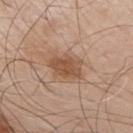| feature | finding |
|---|---|
| anatomic site | the chest |
| subject | male, aged 78 to 82 |
| imaging modality | ~15 mm tile from a whole-body skin photo |